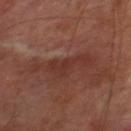Image and clinical context:
Automated image analysis of the tile measured a lesion color around L≈33 a*≈23 b*≈24 in CIELAB and a normalized border contrast of about 6. And it measured a peripheral color-asymmetry measure near 1. And it measured a classifier nevus-likeness of about 0/100 and a detector confidence of about 65 out of 100 that the crop contains a lesion. The lesion is on the right lower leg. Cropped from a whole-body photographic skin survey; the tile spans about 15 mm. Longest diameter approximately 7 mm. A male subject aged approximately 70. This is a cross-polarized tile.